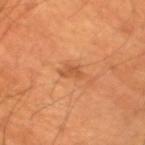Notes:
- workup — total-body-photography surveillance lesion; no biopsy
- site — the right upper arm
- lesion size — ≈3 mm
- tile lighting — cross-polarized illumination
- image source — 15 mm crop, total-body photography
- subject — male, approximately 70 years of age